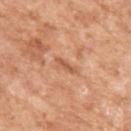| field | value |
|---|---|
| follow-up | total-body-photography surveillance lesion; no biopsy |
| diameter | ~2.5 mm (longest diameter) |
| subject | female, aged approximately 75 |
| illumination | white-light |
| location | the left upper arm |
| image | ~15 mm crop, total-body skin-cancer survey |